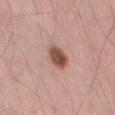Cropped from a total-body skin-imaging series; the visible field is about 15 mm. The tile uses white-light illumination. A male patient aged approximately 55. The lesion is located on the left thigh.